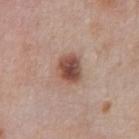Impression:
Part of a total-body skin-imaging series; this lesion was reviewed on a skin check and was not flagged for biopsy.
Context:
On the chest. The tile uses white-light illumination. Measured at roughly 3.5 mm in maximum diameter. The subject is a male approximately 80 years of age. A 15 mm close-up tile from a total-body photography series done for melanoma screening.